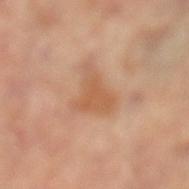Impression:
The lesion was photographed on a routine skin check and not biopsied; there is no pathology result.